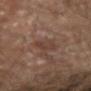workup — total-body-photography surveillance lesion; no biopsy | size — ≈3 mm | lighting — cross-polarized illumination | TBP lesion metrics — an average lesion color of about L≈38 a*≈18 b*≈23 (CIELAB), roughly 6 lightness units darker than nearby skin, and a lesion-to-skin contrast of about 6 (normalized; higher = more distinct); a nevus-likeness score of about 5/100 and a detector confidence of about 100 out of 100 that the crop contains a lesion | patient — male, about 65 years old | anatomic site — the right forearm | image source — total-body-photography crop, ~15 mm field of view.